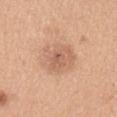Impression:
Recorded during total-body skin imaging; not selected for excision or biopsy.
Clinical summary:
Automated tile analysis of the lesion measured an automated nevus-likeness rating near 5 out of 100 and lesion-presence confidence of about 100/100. A close-up tile cropped from a whole-body skin photograph, about 15 mm across. From the left upper arm. The subject is a female aged 28–32. Imaged with white-light lighting. The recorded lesion diameter is about 5 mm.